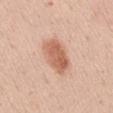<tbp_lesion>
  <biopsy_status>not biopsied; imaged during a skin examination</biopsy_status>
  <site>mid back</site>
  <patient>
    <sex>female</sex>
    <age_approx>40</age_approx>
  </patient>
  <automated_metrics>
    <area_mm2_approx>12.0</area_mm2_approx>
    <shape_asymmetry>0.15</shape_asymmetry>
    <nevus_likeness_0_100>95</nevus_likeness_0_100>
    <lesion_detection_confidence_0_100>100</lesion_detection_confidence_0_100>
  </automated_metrics>
  <image>
    <source>total-body photography crop</source>
    <field_of_view_mm>15</field_of_view_mm>
  </image>
  <lighting>white-light</lighting>
</tbp_lesion>